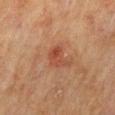{"biopsy_status": "not biopsied; imaged during a skin examination", "patient": {"sex": "male", "age_approx": 70}, "image": {"source": "total-body photography crop", "field_of_view_mm": 15}, "site": "mid back", "lighting": "cross-polarized"}